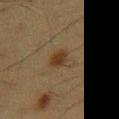Recorded during total-body skin imaging; not selected for excision or biopsy. The total-body-photography lesion software estimated an average lesion color of about L≈28 a*≈12 b*≈25 (CIELAB). It also reported an automated nevus-likeness rating near 95 out of 100. The tile uses cross-polarized illumination. From the mid back. A close-up tile cropped from a whole-body skin photograph, about 15 mm across. About 2.5 mm across. A male subject, approximately 60 years of age.